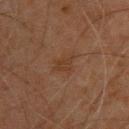{"biopsy_status": "not biopsied; imaged during a skin examination", "site": "upper back", "patient": {"sex": "male", "age_approx": 45}, "image": {"source": "total-body photography crop", "field_of_view_mm": 15}}